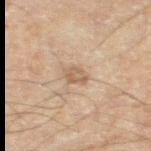<tbp_lesion>
  <patient>
    <sex>male</sex>
    <age_approx>60</age_approx>
  </patient>
  <lesion_size>
    <long_diameter_mm_approx>2.5</long_diameter_mm_approx>
  </lesion_size>
  <image>
    <source>total-body photography crop</source>
    <field_of_view_mm>15</field_of_view_mm>
  </image>
  <site>left thigh</site>
  <automated_metrics>
    <cielab_L>44</cielab_L>
    <cielab_a>12</cielab_a>
    <cielab_b>24</cielab_b>
    <vs_skin_darker_L>7.0</vs_skin_darker_L>
    <vs_skin_contrast_norm>6.0</vs_skin_contrast_norm>
  </automated_metrics>
</tbp_lesion>